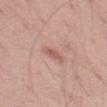Longest diameter approximately 3 mm. Captured under white-light illumination. On the left thigh. A lesion tile, about 15 mm wide, cut from a 3D total-body photograph. A male patient, roughly 50 years of age.